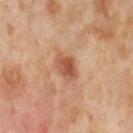Q: Was a biopsy performed?
A: no biopsy performed (imaged during a skin exam)
Q: What is the imaging modality?
A: total-body-photography crop, ~15 mm field of view
Q: Automated lesion metrics?
A: an area of roughly 5.5 mm², an eccentricity of roughly 0.7, and a shape-asymmetry score of about 0.2 (0 = symmetric); an average lesion color of about L≈54 a*≈26 b*≈34 (CIELAB) and roughly 12 lightness units darker than nearby skin; internal color variation of about 4.5 on a 0–10 scale
Q: Where on the body is the lesion?
A: the right thigh
Q: How was the tile lit?
A: cross-polarized illumination
Q: Who is the patient?
A: female, aged 53 to 57